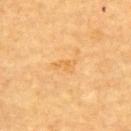Case summary:
- biopsy status — catalogued during a skin exam; not biopsied
- diameter — about 3 mm
- patient — aged 58–62
- acquisition — ~15 mm crop, total-body skin-cancer survey
- automated metrics — an area of roughly 3 mm² and an eccentricity of roughly 0.85
- body site — the back